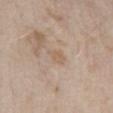Assessment:
This lesion was catalogued during total-body skin photography and was not selected for biopsy.
Acquisition and patient details:
From the front of the torso. A roughly 15 mm field-of-view crop from a total-body skin photograph. Longest diameter approximately 2.5 mm. A female patient, about 30 years old. This is a white-light tile.